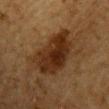Recorded during total-body skin imaging; not selected for excision or biopsy.
A male patient, aged 83 or older.
The total-body-photography lesion software estimated a shape eccentricity near 0.8.
Approximately 7.5 mm at its widest.
A region of skin cropped from a whole-body photographic capture, roughly 15 mm wide.
The lesion is on the chest.
Captured under cross-polarized illumination.